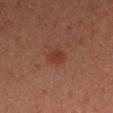follow-up: total-body-photography surveillance lesion; no biopsy | patient: male, aged approximately 45 | diameter: ~2.5 mm (longest diameter) | tile lighting: cross-polarized illumination | location: the right upper arm | imaging modality: total-body-photography crop, ~15 mm field of view.